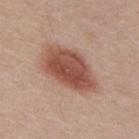Assessment:
Recorded during total-body skin imaging; not selected for excision or biopsy.
Image and clinical context:
A lesion tile, about 15 mm wide, cut from a 3D total-body photograph. The lesion is located on the back. About 7 mm across. A male patient aged 33 to 37. The tile uses white-light illumination.